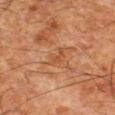Assessment: Part of a total-body skin-imaging series; this lesion was reviewed on a skin check and was not flagged for biopsy. Clinical summary: A close-up tile cropped from a whole-body skin photograph, about 15 mm across. Automated image analysis of the tile measured an area of roughly 3 mm², a shape eccentricity near 0.8, and a symmetry-axis asymmetry near 0.35. It also reported a border-irregularity rating of about 4.5/10, a color-variation rating of about 1/10, and radial color variation of about 0.5. The software also gave an automated nevus-likeness rating near 0 out of 100 and lesion-presence confidence of about 100/100. From the left thigh. Captured under cross-polarized illumination. About 2.5 mm across. A male subject, aged around 80.